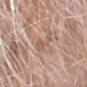Assessment: Imaged during a routine full-body skin examination; the lesion was not biopsied and no histopathology is available. Image and clinical context: On the left upper arm. Automated tile analysis of the lesion measured an area of roughly 5 mm² and two-axis asymmetry of about 0.5. And it measured a border-irregularity index near 6/10, a color-variation rating of about 1/10, and a peripheral color-asymmetry measure near 0.5. It also reported a nevus-likeness score of about 0/100. A 15 mm close-up extracted from a 3D total-body photography capture. The subject is a male roughly 75 years of age. Imaged with white-light lighting. The lesion's longest dimension is about 4.5 mm.